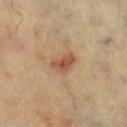Clinical impression: Recorded during total-body skin imaging; not selected for excision or biopsy. Clinical summary: From the right lower leg. A 15 mm close-up extracted from a 3D total-body photography capture. The subject is about 60 years old. Approximately 3.5 mm at its widest.